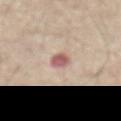biopsy status = catalogued during a skin exam; not biopsied
image source = ~15 mm tile from a whole-body skin photo
body site = the abdomen
automated lesion analysis = a mean CIELAB color near L≈59 a*≈22 b*≈23, about 13 CIELAB-L* units darker than the surrounding skin, and a normalized border contrast of about 9; border irregularity of about 2 on a 0–10 scale, a within-lesion color-variation index near 3/10, and peripheral color asymmetry of about 1
size = about 2.5 mm
patient = male, roughly 65 years of age